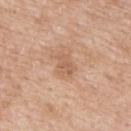Assessment:
The lesion was tiled from a total-body skin photograph and was not biopsied.